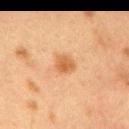biopsy status: total-body-photography surveillance lesion; no biopsy | imaging modality: 15 mm crop, total-body photography | site: the back | illumination: cross-polarized illumination | subject: female, aged 38–42 | automated metrics: an average lesion color of about L≈51 a*≈20 b*≈37 (CIELAB), about 9 CIELAB-L* units darker than the surrounding skin, and a lesion-to-skin contrast of about 7.5 (normalized; higher = more distinct) | size: ~2.5 mm (longest diameter).